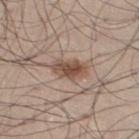The lesion was tiled from a total-body skin photograph and was not biopsied.
A male subject, approximately 30 years of age.
This is a white-light tile.
A 15 mm crop from a total-body photograph taken for skin-cancer surveillance.
From the leg.